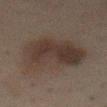Impression:
Imaged during a routine full-body skin examination; the lesion was not biopsied and no histopathology is available.
Image and clinical context:
The lesion is located on the front of the torso. This image is a 15 mm lesion crop taken from a total-body photograph. The subject is a male in their 50s.